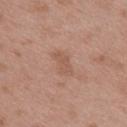Impression:
Imaged during a routine full-body skin examination; the lesion was not biopsied and no histopathology is available.
Acquisition and patient details:
The lesion-visualizer software estimated an area of roughly 5 mm², a shape eccentricity near 0.9, and a symmetry-axis asymmetry near 0.35. The software also gave an average lesion color of about L≈55 a*≈20 b*≈29 (CIELAB) and about 7 CIELAB-L* units darker than the surrounding skin. It also reported a classifier nevus-likeness of about 0/100 and a detector confidence of about 100 out of 100 that the crop contains a lesion. A female subject about 40 years old. The recorded lesion diameter is about 3.5 mm. The lesion is located on the back. A close-up tile cropped from a whole-body skin photograph, about 15 mm across. Captured under white-light illumination.